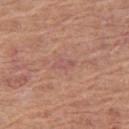notes = no biopsy performed (imaged during a skin exam)
image-analysis metrics = a footprint of about 3 mm², a shape eccentricity near 0.9, and two-axis asymmetry of about 0.3; a mean CIELAB color near L≈55 a*≈23 b*≈25 and a lesion–skin lightness drop of about 5; a nevus-likeness score of about 0/100 and lesion-presence confidence of about 95/100
location = the leg
patient = female, aged 53 to 57
acquisition = 15 mm crop, total-body photography
diameter = about 3 mm